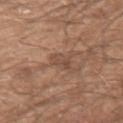Part of a total-body skin-imaging series; this lesion was reviewed on a skin check and was not flagged for biopsy. The total-body-photography lesion software estimated a classifier nevus-likeness of about 0/100 and a detector confidence of about 90 out of 100 that the crop contains a lesion. The lesion is on the left forearm. The subject is a male about 50 years old. A 15 mm close-up extracted from a 3D total-body photography capture. Captured under white-light illumination. About 3 mm across.